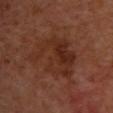workup = total-body-photography surveillance lesion; no biopsy | TBP lesion metrics = a mean CIELAB color near L≈29 a*≈22 b*≈27, a lesion–skin lightness drop of about 6, and a normalized border contrast of about 6.5; a border-irregularity rating of about 7/10, a within-lesion color-variation index near 5/10, and a peripheral color-asymmetry measure near 2; a classifier nevus-likeness of about 0/100 and a detector confidence of about 100 out of 100 that the crop contains a lesion | size = about 7.5 mm | subject = female, aged 38 to 42 | image source = 15 mm crop, total-body photography | tile lighting = cross-polarized illumination | anatomic site = the back.